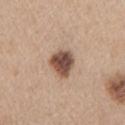Captured during whole-body skin photography for melanoma surveillance; the lesion was not biopsied. The total-body-photography lesion software estimated a lesion area of about 8.5 mm² and a shape-asymmetry score of about 0.25 (0 = symmetric). It also reported a lesion color around L≈49 a*≈18 b*≈27 in CIELAB, roughly 18 lightness units darker than nearby skin, and a normalized lesion–skin contrast near 12. The software also gave a border-irregularity rating of about 2/10 and internal color variation of about 5.5 on a 0–10 scale. The patient is a female in their mid- to late 40s. This image is a 15 mm lesion crop taken from a total-body photograph. Measured at roughly 3.5 mm in maximum diameter. On the left upper arm. Captured under white-light illumination.